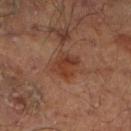- workup: no biopsy performed (imaged during a skin exam)
- lighting: cross-polarized illumination
- imaging modality: ~15 mm crop, total-body skin-cancer survey
- patient: male, in their mid- to late 60s
- site: the right lower leg